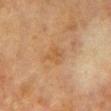Assessment:
This lesion was catalogued during total-body skin photography and was not selected for biopsy.
Context:
Captured under cross-polarized illumination. The lesion-visualizer software estimated a footprint of about 6 mm², a shape eccentricity near 0.7, and a shape-asymmetry score of about 0.3 (0 = symmetric). And it measured an automated nevus-likeness rating near 0 out of 100 and a lesion-detection confidence of about 100/100. A lesion tile, about 15 mm wide, cut from a 3D total-body photograph. A female subject, in their mid-50s. The recorded lesion diameter is about 3.5 mm. From the chest.